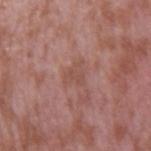Part of a total-body skin-imaging series; this lesion was reviewed on a skin check and was not flagged for biopsy. A male subject aged around 40. Located on the right upper arm. A 15 mm crop from a total-body photograph taken for skin-cancer surveillance.